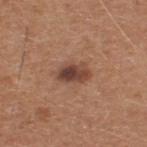Assessment:
The lesion was photographed on a routine skin check and not biopsied; there is no pathology result.
Image and clinical context:
This is a white-light tile. The lesion's longest dimension is about 3.5 mm. The lesion is on the upper back. A male patient aged 63–67. Cropped from a total-body skin-imaging series; the visible field is about 15 mm. Automated image analysis of the tile measured a mean CIELAB color near L≈43 a*≈20 b*≈27, a lesion–skin lightness drop of about 12, and a normalized border contrast of about 9.5. The analysis additionally found border irregularity of about 2.5 on a 0–10 scale, internal color variation of about 6 on a 0–10 scale, and peripheral color asymmetry of about 2. The software also gave a classifier nevus-likeness of about 80/100 and a detector confidence of about 100 out of 100 that the crop contains a lesion.